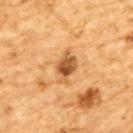notes=imaged on a skin check; not biopsied | tile lighting=cross-polarized illumination | acquisition=15 mm crop, total-body photography | lesion diameter=≈4 mm | patient=male, roughly 85 years of age or older | body site=the upper back.